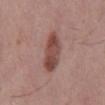Context: About 5 mm across. The tile uses white-light illumination. A male subject, roughly 55 years of age. A 15 mm close-up tile from a total-body photography series done for melanoma screening. Automated image analysis of the tile measured a border-irregularity index near 2/10 and peripheral color asymmetry of about 1. Located on the mid back.